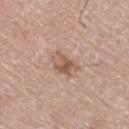Impression:
Recorded during total-body skin imaging; not selected for excision or biopsy.
Acquisition and patient details:
The total-body-photography lesion software estimated an average lesion color of about L≈57 a*≈19 b*≈28 (CIELAB), roughly 10 lightness units darker than nearby skin, and a normalized border contrast of about 7. The software also gave border irregularity of about 3.5 on a 0–10 scale and peripheral color asymmetry of about 2. The analysis additionally found a classifier nevus-likeness of about 50/100 and a lesion-detection confidence of about 100/100. Located on the right thigh. This is a white-light tile. Approximately 4 mm at its widest. A male subject, aged around 75. A 15 mm close-up extracted from a 3D total-body photography capture.